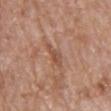Impression:
This lesion was catalogued during total-body skin photography and was not selected for biopsy.
Context:
Located on the back. The tile uses white-light illumination. A female patient, aged around 85 or older. The lesion-visualizer software estimated a border-irregularity rating of about 4.5/10, internal color variation of about 1.5 on a 0–10 scale, and peripheral color asymmetry of about 0.5. It also reported a classifier nevus-likeness of about 0/100 and a lesion-detection confidence of about 70/100. The lesion's longest dimension is about 3.5 mm. A 15 mm crop from a total-body photograph taken for skin-cancer surveillance.